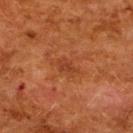Part of a total-body skin-imaging series; this lesion was reviewed on a skin check and was not flagged for biopsy.
A female patient aged approximately 50.
The lesion is on the upper back.
A 15 mm crop from a total-body photograph taken for skin-cancer surveillance.